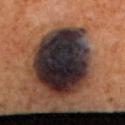biopsy status = no biopsy performed (imaged during a skin exam)
TBP lesion metrics = about 24 CIELAB-L* units darker than the surrounding skin and a normalized lesion–skin contrast near 24.5; a border-irregularity rating of about 1/10 and radial color variation of about 2.5
anatomic site = the back
image = 15 mm crop, total-body photography
illumination = cross-polarized
patient = female, aged approximately 40
diameter = ≈8.5 mm